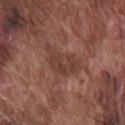A lesion tile, about 15 mm wide, cut from a 3D total-body photograph.
A male patient in their mid- to late 70s.
The lesion is on the chest.
The recorded lesion diameter is about 5 mm.